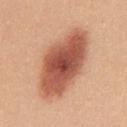{"biopsy_status": "not biopsied; imaged during a skin examination", "patient": {"sex": "female", "age_approx": 25}, "lighting": "white-light", "image": {"source": "total-body photography crop", "field_of_view_mm": 15}, "site": "chest", "lesion_size": {"long_diameter_mm_approx": 10.0}}